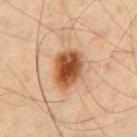The lesion was photographed on a routine skin check and not biopsied; there is no pathology result. A male patient, in their mid-40s. An algorithmic analysis of the crop reported a footprint of about 12 mm², a shape eccentricity near 0.7, and a symmetry-axis asymmetry near 0.2. It also reported a border-irregularity index near 2/10, internal color variation of about 6.5 on a 0–10 scale, and radial color variation of about 1.5. From the chest. A lesion tile, about 15 mm wide, cut from a 3D total-body photograph.